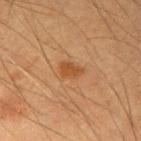Impression: Part of a total-body skin-imaging series; this lesion was reviewed on a skin check and was not flagged for biopsy.